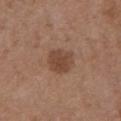The lesion was photographed on a routine skin check and not biopsied; there is no pathology result. Located on the chest. Measured at roughly 3.5 mm in maximum diameter. The subject is a female aged 63–67. Cropped from a whole-body photographic skin survey; the tile spans about 15 mm. Automated image analysis of the tile measured a classifier nevus-likeness of about 60/100 and a detector confidence of about 100 out of 100 that the crop contains a lesion.